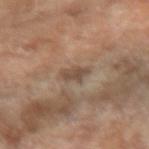notes: catalogued during a skin exam; not biopsied
imaging modality: ~15 mm crop, total-body skin-cancer survey
illumination: cross-polarized
location: the left forearm
patient: female, in their 70s
image-analysis metrics: a footprint of about 4 mm² and an eccentricity of roughly 0.85; border irregularity of about 4 on a 0–10 scale and a color-variation rating of about 1.5/10